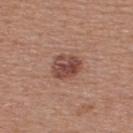Imaged during a routine full-body skin examination; the lesion was not biopsied and no histopathology is available.
A close-up tile cropped from a whole-body skin photograph, about 15 mm across.
A male subject in their mid-50s.
Located on the upper back.